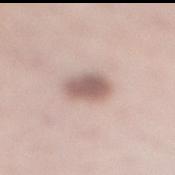This lesion was catalogued during total-body skin photography and was not selected for biopsy.
Approximately 3.5 mm at its widest.
Cropped from a total-body skin-imaging series; the visible field is about 15 mm.
A female subject approximately 45 years of age.
This is a white-light tile.
The total-body-photography lesion software estimated a lesion color around L≈59 a*≈17 b*≈21 in CIELAB, a lesion–skin lightness drop of about 14, and a lesion-to-skin contrast of about 8.5 (normalized; higher = more distinct). The analysis additionally found an automated nevus-likeness rating near 65 out of 100 and a lesion-detection confidence of about 100/100.
The lesion is on the leg.